The lesion is located on the right lower leg · Automated tile analysis of the lesion measured a lesion color around L≈41 a*≈20 b*≈23 in CIELAB and about 7 CIELAB-L* units darker than the surrounding skin. It also reported border irregularity of about 8.5 on a 0–10 scale, internal color variation of about 2 on a 0–10 scale, and radial color variation of about 1. The analysis additionally found a classifier nevus-likeness of about 0/100 · a male subject aged around 60 · approximately 5.5 mm at its widest · the tile uses cross-polarized illumination · cropped from a whole-body photographic skin survey; the tile spans about 15 mm:
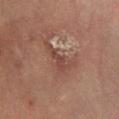diagnosis:
  histopathology: actinic keratosis
  malignancy: indeterminate
  taxonomic_path:
    - Indeterminate
    - Indeterminate epidermal proliferations
    - Solar or actinic keratosis